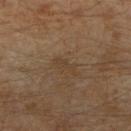No biopsy was performed on this lesion — it was imaged during a full skin examination and was not determined to be concerning. The lesion's longest dimension is about 3 mm. The lesion is on the left leg. A close-up tile cropped from a whole-body skin photograph, about 15 mm across. This is a cross-polarized tile. A male patient, aged around 30.